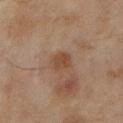This lesion was catalogued during total-body skin photography and was not selected for biopsy.
Cropped from a total-body skin-imaging series; the visible field is about 15 mm.
The total-body-photography lesion software estimated a footprint of about 4.5 mm², a shape eccentricity near 0.6, and a shape-asymmetry score of about 0.25 (0 = symmetric). It also reported a border-irregularity rating of about 2/10 and peripheral color asymmetry of about 1. It also reported an automated nevus-likeness rating near 0 out of 100 and a detector confidence of about 100 out of 100 that the crop contains a lesion.
Measured at roughly 2.5 mm in maximum diameter.
The lesion is on the left lower leg.
A female patient aged 58–62.
Captured under cross-polarized illumination.